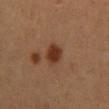Part of a total-body skin-imaging series; this lesion was reviewed on a skin check and was not flagged for biopsy. The recorded lesion diameter is about 3.5 mm. A region of skin cropped from a whole-body photographic capture, roughly 15 mm wide. A female subject. The lesion-visualizer software estimated a footprint of about 6 mm² and a shape-asymmetry score of about 0.2 (0 = symmetric). From the mid back. Imaged with cross-polarized lighting.